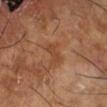biopsy_status: not biopsied; imaged during a skin examination
automated_metrics:
  area_mm2_approx: 3.5
  eccentricity: 0.9
  shape_asymmetry: 0.3
  cielab_L: 44
  cielab_a: 23
  cielab_b: 33
  vs_skin_darker_L: 7.0
  vs_skin_contrast_norm: 5.5
patient:
  sex: male
  age_approx: 65
site: right lower leg
image:
  source: total-body photography crop
  field_of_view_mm: 15
lesion_size:
  long_diameter_mm_approx: 3.0
lighting: cross-polarized